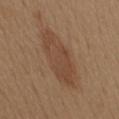Clinical impression: The lesion was photographed on a routine skin check and not biopsied; there is no pathology result. Context: A region of skin cropped from a whole-body photographic capture, roughly 15 mm wide. The subject is a female aged around 40. From the mid back.